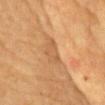Q: What is the imaging modality?
A: ~15 mm crop, total-body skin-cancer survey
Q: Who is the patient?
A: male, aged 83 or over
Q: How was the tile lit?
A: cross-polarized illumination
Q: What did automated image analysis measure?
A: a lesion area of about 6 mm²; a classifier nevus-likeness of about 0/100 and a detector confidence of about 60 out of 100 that the crop contains a lesion
Q: What is the lesion's diameter?
A: about 4 mm
Q: What is the anatomic site?
A: the chest